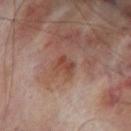Clinical impression: This lesion was catalogued during total-body skin photography and was not selected for biopsy. Acquisition and patient details: A male subject, approximately 70 years of age. This is a cross-polarized tile. Longest diameter approximately 2.5 mm. The total-body-photography lesion software estimated a lesion area of about 4 mm², an outline eccentricity of about 0.6 (0 = round, 1 = elongated), and two-axis asymmetry of about 0.25. Located on the left thigh. A lesion tile, about 15 mm wide, cut from a 3D total-body photograph.